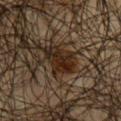Image and clinical context:
Located on the mid back. An algorithmic analysis of the crop reported a lesion area of about 7 mm², an eccentricity of roughly 0.9, and two-axis asymmetry of about 0.25. It also reported an average lesion color of about L≈22 a*≈15 b*≈23 (CIELAB), roughly 10 lightness units darker than nearby skin, and a normalized border contrast of about 11.5. The recorded lesion diameter is about 4.5 mm. Captured under cross-polarized illumination. This image is a 15 mm lesion crop taken from a total-body photograph. A male patient aged 63 to 67.